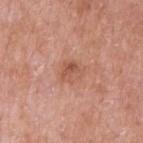Findings:
* follow-up: imaged on a skin check; not biopsied
* imaging modality: 15 mm crop, total-body photography
* site: the left upper arm
* subject: male, about 60 years old